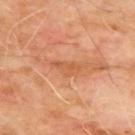Assessment: The lesion was tiled from a total-body skin photograph and was not biopsied.